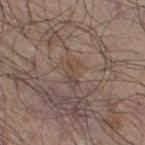The recorded lesion diameter is about 3 mm. This is a white-light tile. Cropped from a total-body skin-imaging series; the visible field is about 15 mm. A male subject, in their 50s. From the right thigh.